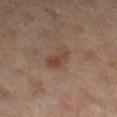workup — imaged on a skin check; not biopsied.